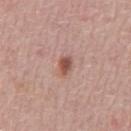The lesion was tiled from a total-body skin photograph and was not biopsied. A male subject, about 70 years old. Automated image analysis of the tile measured an eccentricity of roughly 0.8 and two-axis asymmetry of about 0.35. And it measured a lesion color around L≈52 a*≈23 b*≈26 in CIELAB. About 2.5 mm across. Captured under white-light illumination. A lesion tile, about 15 mm wide, cut from a 3D total-body photograph. The lesion is on the chest.